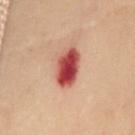No biopsy was performed on this lesion — it was imaged during a full skin examination and was not determined to be concerning. The lesion-visualizer software estimated a footprint of about 11 mm², a shape eccentricity near 0.8, and two-axis asymmetry of about 0.1. The software also gave lesion-presence confidence of about 100/100. A female subject in their 60s. A 15 mm close-up extracted from a 3D total-body photography capture. On the front of the torso. Imaged with cross-polarized lighting.